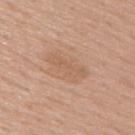Clinical impression:
This lesion was catalogued during total-body skin photography and was not selected for biopsy.
Background:
A male subject, about 55 years old. The lesion is located on the back. A 15 mm close-up tile from a total-body photography series done for melanoma screening.